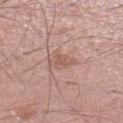Impression: This lesion was catalogued during total-body skin photography and was not selected for biopsy. Context: The subject is a male in their mid-50s. The lesion is located on the left lower leg. Cropped from a total-body skin-imaging series; the visible field is about 15 mm.